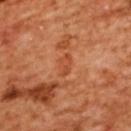The lesion was tiled from a total-body skin photograph and was not biopsied.
A 15 mm close-up tile from a total-body photography series done for melanoma screening.
From the back.
Automated tile analysis of the lesion measured a footprint of about 4 mm² and an eccentricity of roughly 0.85. It also reported a border-irregularity index near 2.5/10 and radial color variation of about 1.
A female subject, aged 53–57.
Longest diameter approximately 3 mm.
The tile uses cross-polarized illumination.